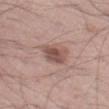Imaged with white-light lighting.
From the left thigh.
A male subject about 55 years old.
A 15 mm close-up extracted from a 3D total-body photography capture.
The total-body-photography lesion software estimated a mean CIELAB color near L≈50 a*≈20 b*≈23, roughly 12 lightness units darker than nearby skin, and a normalized lesion–skin contrast near 8.5. The analysis additionally found border irregularity of about 2.5 on a 0–10 scale, a color-variation rating of about 3/10, and peripheral color asymmetry of about 1.
Approximately 3.5 mm at its widest.